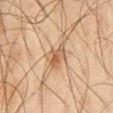{
  "biopsy_status": "not biopsied; imaged during a skin examination",
  "lighting": "cross-polarized",
  "patient": {
    "sex": "male",
    "age_approx": 45
  },
  "lesion_size": {
    "long_diameter_mm_approx": 3.5
  },
  "site": "left thigh",
  "image": {
    "source": "total-body photography crop",
    "field_of_view_mm": 15
  },
  "automated_metrics": {
    "eccentricity": 0.85,
    "shape_asymmetry": 0.35,
    "nevus_likeness_0_100": 15,
    "lesion_detection_confidence_0_100": 100
  }
}